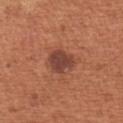Imaged during a routine full-body skin examination; the lesion was not biopsied and no histopathology is available. The lesion-visualizer software estimated a lesion area of about 7 mm², an eccentricity of roughly 0.25, and a shape-asymmetry score of about 0.25 (0 = symmetric). The analysis additionally found a mean CIELAB color near L≈43 a*≈25 b*≈27, a lesion–skin lightness drop of about 11, and a normalized border contrast of about 9.5. The software also gave an automated nevus-likeness rating near 90 out of 100 and lesion-presence confidence of about 100/100. A female subject, about 65 years old. The lesion's longest dimension is about 3 mm. From the left upper arm. Cropped from a total-body skin-imaging series; the visible field is about 15 mm.